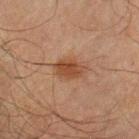follow-up: imaged on a skin check; not biopsied | lesion size: ≈3.5 mm | patient: male, aged approximately 70 | body site: the left upper arm | tile lighting: cross-polarized | image source: ~15 mm crop, total-body skin-cancer survey.